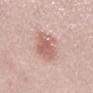follow-up = catalogued during a skin exam; not biopsied
size = ≈5.5 mm
illumination = white-light illumination
subject = male, roughly 25 years of age
location = the back
acquisition = total-body-photography crop, ~15 mm field of view